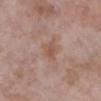Notes:
* workup · imaged on a skin check; not biopsied
* site · the right lower leg
* image · ~15 mm crop, total-body skin-cancer survey
* tile lighting · white-light
* automated metrics · an area of roughly 5 mm², a shape eccentricity near 0.6, and two-axis asymmetry of about 0.35; an average lesion color of about L≈54 a*≈19 b*≈27 (CIELAB) and a normalized border contrast of about 5.5; a classifier nevus-likeness of about 0/100 and a detector confidence of about 100 out of 100 that the crop contains a lesion
* subject · female, aged around 70
* lesion diameter · ~3 mm (longest diameter)